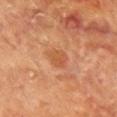workup = imaged on a skin check; not biopsied